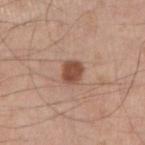The patient is a male aged 53–57.
The recorded lesion diameter is about 3 mm.
An algorithmic analysis of the crop reported an area of roughly 5.5 mm² and two-axis asymmetry of about 0.2. The analysis additionally found a mean CIELAB color near L≈49 a*≈22 b*≈29.
Imaged with white-light lighting.
A close-up tile cropped from a whole-body skin photograph, about 15 mm across.
From the arm.